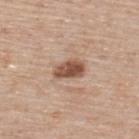notes: catalogued during a skin exam; not biopsied | body site: the upper back | image-analysis metrics: an area of roughly 6.5 mm², an outline eccentricity of about 0.75 (0 = round, 1 = elongated), and a symmetry-axis asymmetry near 0.2; a mean CIELAB color near L≈52 a*≈21 b*≈29; a border-irregularity rating of about 2/10, a within-lesion color-variation index near 4/10, and radial color variation of about 1.5; lesion-presence confidence of about 100/100 | acquisition: 15 mm crop, total-body photography | subject: male, aged around 75 | size: ≈3.5 mm.